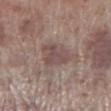follow-up=total-body-photography surveillance lesion; no biopsy.